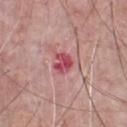Captured during whole-body skin photography for melanoma surveillance; the lesion was not biopsied. A region of skin cropped from a whole-body photographic capture, roughly 15 mm wide. A male patient aged 68 to 72. On the chest.